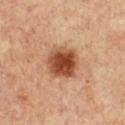workup: total-body-photography surveillance lesion; no biopsy
size: ~4 mm (longest diameter)
patient: female, about 45 years old
acquisition: ~15 mm tile from a whole-body skin photo
automated metrics: a footprint of about 13 mm² and a shape-asymmetry score of about 0.15 (0 = symmetric); a detector confidence of about 100 out of 100 that the crop contains a lesion
site: the chest